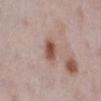{
  "biopsy_status": "not biopsied; imaged during a skin examination",
  "site": "right lower leg",
  "patient": {
    "sex": "female",
    "age_approx": 30
  },
  "image": {
    "source": "total-body photography crop",
    "field_of_view_mm": 15
  }
}